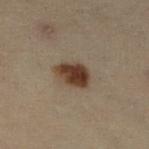Assessment: The lesion was tiled from a total-body skin photograph and was not biopsied. Acquisition and patient details: The lesion is on the leg. This is a cross-polarized tile. A female subject, about 50 years old. Cropped from a total-body skin-imaging series; the visible field is about 15 mm.